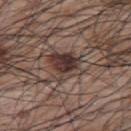Case summary:
– notes: imaged on a skin check; not biopsied
– site: the back
– automated metrics: a lesion color around L≈36 a*≈16 b*≈20 in CIELAB, about 13 CIELAB-L* units darker than the surrounding skin, and a normalized border contrast of about 11; border irregularity of about 3.5 on a 0–10 scale, a within-lesion color-variation index near 6.5/10, and radial color variation of about 2
– diameter: about 4 mm
– illumination: white-light
– imaging modality: ~15 mm crop, total-body skin-cancer survey
– patient: male, approximately 70 years of age